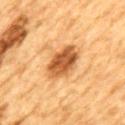Located on the mid back. Automated tile analysis of the lesion measured a footprint of about 11 mm², an eccentricity of roughly 0.85, and a shape-asymmetry score of about 0.15 (0 = symmetric). It also reported an average lesion color of about L≈49 a*≈24 b*≈40 (CIELAB), about 16 CIELAB-L* units darker than the surrounding skin, and a lesion-to-skin contrast of about 10.5 (normalized; higher = more distinct). It also reported an automated nevus-likeness rating near 80 out of 100 and a lesion-detection confidence of about 100/100. A lesion tile, about 15 mm wide, cut from a 3D total-body photograph. Longest diameter approximately 5 mm. A male subject roughly 85 years of age or older. Captured under cross-polarized illumination.